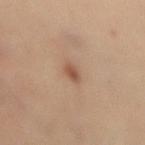Captured during whole-body skin photography for melanoma surveillance; the lesion was not biopsied. A 15 mm crop from a total-body photograph taken for skin-cancer surveillance. A female patient aged 43 to 47. The lesion is located on the mid back.